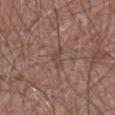Captured during whole-body skin photography for melanoma surveillance; the lesion was not biopsied.
A male patient, aged around 50.
Longest diameter approximately 3 mm.
From the right forearm.
A lesion tile, about 15 mm wide, cut from a 3D total-body photograph.
The total-body-photography lesion software estimated an area of roughly 3 mm² and a symmetry-axis asymmetry near 0.45. And it measured a color-variation rating of about 0/10. And it measured an automated nevus-likeness rating near 0 out of 100 and lesion-presence confidence of about 80/100.
Imaged with white-light lighting.